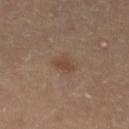• biopsy status: imaged on a skin check; not biopsied
• patient: female, in their mid- to late 50s
• site: the left lower leg
• image-analysis metrics: a lesion area of about 2.5 mm² and a shape-asymmetry score of about 0.3 (0 = symmetric)
• image source: ~15 mm tile from a whole-body skin photo
• lesion diameter: about 2 mm
• lighting: cross-polarized illumination